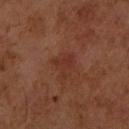Recorded during total-body skin imaging; not selected for excision or biopsy. A roughly 15 mm field-of-view crop from a total-body skin photograph. Imaged with cross-polarized lighting. Located on the right upper arm. A female subject, roughly 60 years of age. Approximately 3 mm at its widest. Automated tile analysis of the lesion measured two-axis asymmetry of about 0.55. The analysis additionally found a mean CIELAB color near L≈28 a*≈22 b*≈25, roughly 5 lightness units darker than nearby skin, and a normalized border contrast of about 5. It also reported a border-irregularity index near 6/10, internal color variation of about 2 on a 0–10 scale, and peripheral color asymmetry of about 0.5.